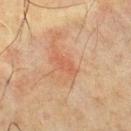image source: ~15 mm tile from a whole-body skin photo | site: the chest | subject: male, aged approximately 70 | size: about 3.5 mm | automated metrics: an average lesion color of about L≈48 a*≈22 b*≈30 (CIELAB), roughly 6 lightness units darker than nearby skin, and a normalized lesion–skin contrast near 4.5; a lesion-detection confidence of about 100/100.